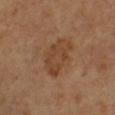The lesion was photographed on a routine skin check and not biopsied; there is no pathology result. A female patient, in their mid- to late 60s. A roughly 15 mm field-of-view crop from a total-body skin photograph. The lesion is on the right lower leg.